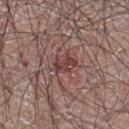Part of a total-body skin-imaging series; this lesion was reviewed on a skin check and was not flagged for biopsy. Approximately 3 mm at its widest. A male patient, aged 63–67. The total-body-photography lesion software estimated a lesion color around L≈41 a*≈23 b*≈20 in CIELAB, roughly 9 lightness units darker than nearby skin, and a normalized border contrast of about 7.5. A 15 mm crop from a total-body photograph taken for skin-cancer surveillance. The lesion is on the leg. This is a white-light tile.